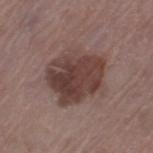biopsy_status: not biopsied; imaged during a skin examination
automated_metrics:
  cielab_L: 40
  cielab_a: 18
  cielab_b: 20
  vs_skin_darker_L: 11.0
  vs_skin_contrast_norm: 9.5
  color_variation_0_10: 5.0
image:
  source: total-body photography crop
  field_of_view_mm: 15
patient:
  sex: female
  age_approx: 70
lesion_size:
  long_diameter_mm_approx: 6.5
site: left thigh
lighting: white-light